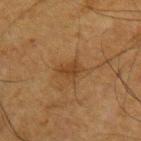biopsy_status: not biopsied; imaged during a skin examination
image:
  source: total-body photography crop
  field_of_view_mm: 15
patient:
  sex: male
  age_approx: 65
site: arm
lighting: cross-polarized
automated_metrics:
  area_mm2_approx: 4.0
  eccentricity: 0.7
  nevus_likeness_0_100: 10
  lesion_detection_confidence_0_100: 100
lesion_size:
  long_diameter_mm_approx: 3.0